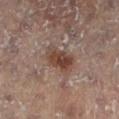workup=total-body-photography surveillance lesion; no biopsy | location=the right leg | image source=~15 mm crop, total-body skin-cancer survey | lighting=cross-polarized illumination | patient=female, in their 80s.